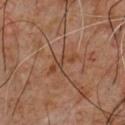No biopsy was performed on this lesion — it was imaged during a full skin examination and was not determined to be concerning. The lesion is on the chest. A close-up tile cropped from a whole-body skin photograph, about 15 mm across. A male subject approximately 60 years of age. Captured under cross-polarized illumination.